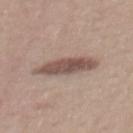notes = imaged on a skin check; not biopsied
subject = female, in their mid-50s
imaging modality = ~15 mm tile from a whole-body skin photo
lesion diameter = ~6 mm (longest diameter)
lighting = white-light illumination
body site = the mid back